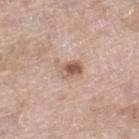The lesion is located on the right lower leg. A region of skin cropped from a whole-body photographic capture, roughly 15 mm wide. Imaged with white-light lighting. A female patient aged approximately 75. The recorded lesion diameter is about 3 mm. Automated tile analysis of the lesion measured a shape eccentricity near 0.8. It also reported about 12 CIELAB-L* units darker than the surrounding skin and a normalized lesion–skin contrast near 7.5. It also reported a border-irregularity rating of about 4/10, a color-variation rating of about 7/10, and radial color variation of about 2.5.